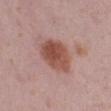Captured during whole-body skin photography for melanoma surveillance; the lesion was not biopsied. About 5 mm across. Imaged with white-light lighting. A female patient about 40 years old. A lesion tile, about 15 mm wide, cut from a 3D total-body photograph. From the left lower leg. Automated image analysis of the tile measured a footprint of about 14 mm², an outline eccentricity of about 0.65 (0 = round, 1 = elongated), and a symmetry-axis asymmetry near 0.15. The software also gave a mean CIELAB color near L≈50 a*≈24 b*≈26, a lesion–skin lightness drop of about 12, and a lesion-to-skin contrast of about 9 (normalized; higher = more distinct). The software also gave border irregularity of about 1.5 on a 0–10 scale, internal color variation of about 4.5 on a 0–10 scale, and peripheral color asymmetry of about 1.5. It also reported an automated nevus-likeness rating near 95 out of 100.